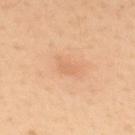biopsy status = no biopsy performed (imaged during a skin exam) | anatomic site = the back | image = 15 mm crop, total-body photography | diameter = about 2.5 mm | patient = male, aged around 30.